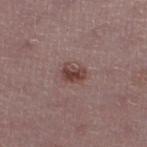The lesion was tiled from a total-body skin photograph and was not biopsied.
A region of skin cropped from a whole-body photographic capture, roughly 15 mm wide.
Approximately 2.5 mm at its widest.
Located on the left lower leg.
The total-body-photography lesion software estimated a border-irregularity rating of about 1.5/10, internal color variation of about 6 on a 0–10 scale, and radial color variation of about 2.
Captured under white-light illumination.
A female patient aged 43 to 47.